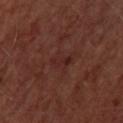Impression: The lesion was photographed on a routine skin check and not biopsied; there is no pathology result. Context: The patient is a female aged around 45. This is a cross-polarized tile. A 15 mm crop from a total-body photograph taken for skin-cancer surveillance. An algorithmic analysis of the crop reported a mean CIELAB color near L≈24 a*≈22 b*≈21, a lesion–skin lightness drop of about 5, and a normalized border contrast of about 5.5. And it measured a border-irregularity index near 3/10, a within-lesion color-variation index near 3/10, and peripheral color asymmetry of about 1. Located on the head or neck. Measured at roughly 3 mm in maximum diameter.